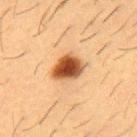{"biopsy_status": "not biopsied; imaged during a skin examination", "lesion_size": {"long_diameter_mm_approx": 3.5}, "patient": {"sex": "male", "age_approx": 55}, "site": "chest", "lighting": "cross-polarized", "automated_metrics": {"cielab_L": 46, "cielab_a": 24, "cielab_b": 36, "vs_skin_darker_L": 19.0}, "image": {"source": "total-body photography crop", "field_of_view_mm": 15}}